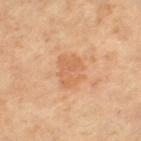biopsy_status: not biopsied; imaged during a skin examination
image:
  source: total-body photography crop
  field_of_view_mm: 15
patient:
  sex: female
  age_approx: 65
site: left thigh